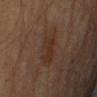The lesion was photographed on a routine skin check and not biopsied; there is no pathology result. A male patient aged approximately 65. A 15 mm crop from a total-body photograph taken for skin-cancer surveillance. Imaged with cross-polarized lighting. From the right upper arm.